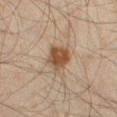The lesion was tiled from a total-body skin photograph and was not biopsied. A 15 mm close-up tile from a total-body photography series done for melanoma screening. The tile uses cross-polarized illumination. A male patient, in their mid- to late 40s. The lesion is located on the left thigh.